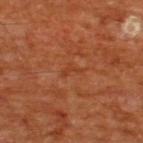notes: imaged on a skin check; not biopsied | automated lesion analysis: an eccentricity of roughly 0.9 and a symmetry-axis asymmetry near 0.6; an average lesion color of about L≈39 a*≈27 b*≈36 (CIELAB); border irregularity of about 6.5 on a 0–10 scale, internal color variation of about 0 on a 0–10 scale, and radial color variation of about 0 | acquisition: ~15 mm crop, total-body skin-cancer survey | subject: male, roughly 65 years of age | site: the upper back.